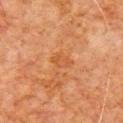Imaged during a routine full-body skin examination; the lesion was not biopsied and no histopathology is available.
A lesion tile, about 15 mm wide, cut from a 3D total-body photograph.
The recorded lesion diameter is about 2.5 mm.
Captured under cross-polarized illumination.
Automated image analysis of the tile measured a shape-asymmetry score of about 0.25 (0 = symmetric). It also reported a lesion color around L≈43 a*≈23 b*≈35 in CIELAB, a lesion–skin lightness drop of about 5, and a lesion-to-skin contrast of about 5 (normalized; higher = more distinct). It also reported border irregularity of about 2.5 on a 0–10 scale, internal color variation of about 1.5 on a 0–10 scale, and a peripheral color-asymmetry measure near 0.5. And it measured a detector confidence of about 100 out of 100 that the crop contains a lesion.
A male subject aged around 80.
The lesion is on the front of the torso.